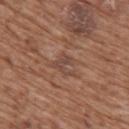follow-up=catalogued during a skin exam; not biopsied
patient=female, aged 73–77
body site=the upper back
tile lighting=white-light illumination
lesion diameter=about 3.5 mm
acquisition=total-body-photography crop, ~15 mm field of view